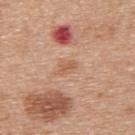<tbp_lesion>
  <biopsy_status>not biopsied; imaged during a skin examination</biopsy_status>
  <site>back</site>
  <lesion_size>
    <long_diameter_mm_approx>2.5</long_diameter_mm_approx>
  </lesion_size>
  <image>
    <source>total-body photography crop</source>
    <field_of_view_mm>15</field_of_view_mm>
  </image>
  <lighting>white-light</lighting>
  <patient>
    <sex>male</sex>
    <age_approx>70</age_approx>
  </patient>
</tbp_lesion>